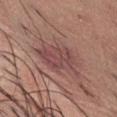Measured at roughly 6 mm in maximum diameter. Cropped from a total-body skin-imaging series; the visible field is about 15 mm. Located on the abdomen. Imaged with white-light lighting. A female patient aged 38 to 42.